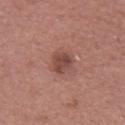Q: Is there a histopathology result?
A: total-body-photography surveillance lesion; no biopsy
Q: Patient demographics?
A: female, about 50 years old
Q: What is the imaging modality?
A: total-body-photography crop, ~15 mm field of view
Q: Lesion location?
A: the left upper arm
Q: What is the lesion's diameter?
A: ~3 mm (longest diameter)
Q: What lighting was used for the tile?
A: white-light